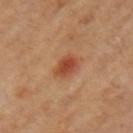workup: imaged on a skin check; not biopsied | subject: female, in their mid- to late 60s | body site: the left upper arm | automated metrics: a lesion area of about 5.5 mm², a shape eccentricity near 0.65, and two-axis asymmetry of about 0.2; a lesion color around L≈46 a*≈27 b*≈34 in CIELAB and roughly 10 lightness units darker than nearby skin; a border-irregularity index near 2/10, internal color variation of about 4 on a 0–10 scale, and peripheral color asymmetry of about 1.5; a nevus-likeness score of about 100/100 | image: ~15 mm crop, total-body skin-cancer survey | lighting: cross-polarized illumination.